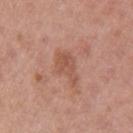follow-up: no biopsy performed (imaged during a skin exam)